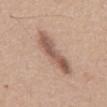Part of a total-body skin-imaging series; this lesion was reviewed on a skin check and was not flagged for biopsy. Automated tile analysis of the lesion measured an eccentricity of roughly 0.95 and a symmetry-axis asymmetry near 0.25. And it measured an average lesion color of about L≈56 a*≈18 b*≈27 (CIELAB), roughly 12 lightness units darker than nearby skin, and a normalized lesion–skin contrast near 8. The lesion is on the mid back. A male subject aged 63 to 67. Cropped from a whole-body photographic skin survey; the tile spans about 15 mm.